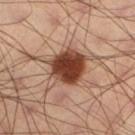Clinical impression: The lesion was tiled from a total-body skin photograph and was not biopsied. Context: The lesion is on the left lower leg. A close-up tile cropped from a whole-body skin photograph, about 15 mm across. Approximately 6.5 mm at its widest. This is a cross-polarized tile. The patient is a male aged around 40.